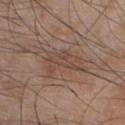biopsy status: total-body-photography surveillance lesion; no biopsy
site: the chest
acquisition: ~15 mm crop, total-body skin-cancer survey
patient: male, in their mid- to late 40s